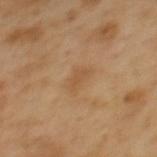{
  "lesion_size": {
    "long_diameter_mm_approx": 3.0
  },
  "site": "mid back",
  "patient": {
    "sex": "male",
    "age_approx": 50
  },
  "image": {
    "source": "total-body photography crop",
    "field_of_view_mm": 15
  },
  "lighting": "cross-polarized"
}